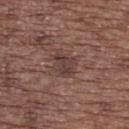Imaged during a routine full-body skin examination; the lesion was not biopsied and no histopathology is available. A 15 mm close-up extracted from a 3D total-body photography capture. Located on the upper back. A female subject aged approximately 75.